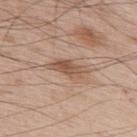Clinical impression: No biopsy was performed on this lesion — it was imaged during a full skin examination and was not determined to be concerning. Background: Captured under white-light illumination. The lesion-visualizer software estimated an area of roughly 7 mm² and a symmetry-axis asymmetry near 0.4. The software also gave an average lesion color of about L≈54 a*≈18 b*≈30 (CIELAB) and a normalized lesion–skin contrast near 7.5. The subject is a male roughly 65 years of age. On the upper back. Measured at roughly 4.5 mm in maximum diameter. A 15 mm close-up tile from a total-body photography series done for melanoma screening.